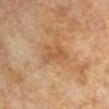Notes:
* notes · total-body-photography surveillance lesion; no biopsy
* lighting · cross-polarized illumination
* body site · the left upper arm
* image source · ~15 mm tile from a whole-body skin photo
* patient · male, in their mid-70s
* lesion size · ~3.5 mm (longest diameter)
* automated metrics · an average lesion color of about L≈44 a*≈18 b*≈31 (CIELAB) and a lesion-to-skin contrast of about 5 (normalized; higher = more distinct); lesion-presence confidence of about 100/100